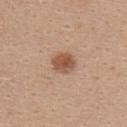Captured during whole-body skin photography for melanoma surveillance; the lesion was not biopsied. The lesion is on the upper back. A female subject approximately 30 years of age. Cropped from a total-body skin-imaging series; the visible field is about 15 mm.